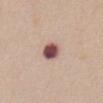anatomic site: the abdomen | subject: female, roughly 55 years of age | image: ~15 mm crop, total-body skin-cancer survey.